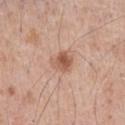biopsy status: total-body-photography surveillance lesion; no biopsy
body site: the chest
lesion diameter: ≈2.5 mm
TBP lesion metrics: an eccentricity of roughly 0.35 and two-axis asymmetry of about 0.25; an average lesion color of about L≈57 a*≈22 b*≈30 (CIELAB), about 11 CIELAB-L* units darker than the surrounding skin, and a lesion-to-skin contrast of about 7.5 (normalized; higher = more distinct); border irregularity of about 2 on a 0–10 scale and internal color variation of about 5 on a 0–10 scale; a classifier nevus-likeness of about 95/100 and lesion-presence confidence of about 100/100
patient: male, in their mid-50s
image source: 15 mm crop, total-body photography
tile lighting: white-light